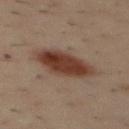Case summary:
* follow-up — imaged on a skin check; not biopsied
* lighting — cross-polarized
* size — ~6 mm (longest diameter)
* patient — male, approximately 40 years of age
* imaging modality — ~15 mm crop, total-body skin-cancer survey
* site — the mid back